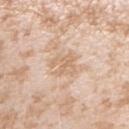<lesion>
<biopsy_status>not biopsied; imaged during a skin examination</biopsy_status>
<lighting>white-light</lighting>
<patient>
  <sex>female</sex>
  <age_approx>25</age_approx>
</patient>
<site>right upper arm</site>
<image>
  <source>total-body photography crop</source>
  <field_of_view_mm>15</field_of_view_mm>
</image>
<lesion_size>
  <long_diameter_mm_approx>3.0</long_diameter_mm_approx>
</lesion_size>
</lesion>